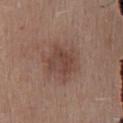notes: imaged on a skin check; not biopsied
anatomic site: the lower back
lesion size: ~3.5 mm (longest diameter)
illumination: white-light illumination
subject: female, aged 38 to 42
imaging modality: 15 mm crop, total-body photography
automated metrics: a mean CIELAB color near L≈43 a*≈19 b*≈24, a lesion–skin lightness drop of about 8, and a lesion-to-skin contrast of about 6.5 (normalized; higher = more distinct)